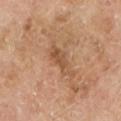No biopsy was performed on this lesion — it was imaged during a full skin examination and was not determined to be concerning. A region of skin cropped from a whole-body photographic capture, roughly 15 mm wide. Automated image analysis of the tile measured a lesion–skin lightness drop of about 9. The software also gave border irregularity of about 5 on a 0–10 scale, a within-lesion color-variation index near 2.5/10, and a peripheral color-asymmetry measure near 0.5. From the leg. About 4.5 mm across. A male subject in their mid- to late 60s. The tile uses cross-polarized illumination.